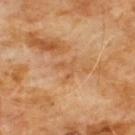Imaged during a routine full-body skin examination; the lesion was not biopsied and no histopathology is available. A male subject, roughly 60 years of age. A roughly 15 mm field-of-view crop from a total-body skin photograph. Longest diameter approximately 2.5 mm. The lesion is on the chest. Imaged with cross-polarized lighting.